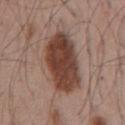{"biopsy_status": "not biopsied; imaged during a skin examination", "image": {"source": "total-body photography crop", "field_of_view_mm": 15}, "site": "chest", "patient": {"sex": "male", "age_approx": 55}}